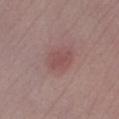No biopsy was performed on this lesion — it was imaged during a full skin examination and was not determined to be concerning. A male subject, aged 48–52. An algorithmic analysis of the crop reported two-axis asymmetry of about 0.3. And it measured border irregularity of about 3.5 on a 0–10 scale, a color-variation rating of about 1/10, and a peripheral color-asymmetry measure near 0.5. It also reported a nevus-likeness score of about 50/100 and a detector confidence of about 100 out of 100 that the crop contains a lesion. Imaged with white-light lighting. A 15 mm close-up extracted from a 3D total-body photography capture. On the right lower leg. Approximately 3 mm at its widest.